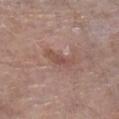Assessment: Captured during whole-body skin photography for melanoma surveillance; the lesion was not biopsied. Clinical summary: The patient is a male about 80 years old. Cropped from a total-body skin-imaging series; the visible field is about 15 mm. On the left lower leg. The lesion's longest dimension is about 3.5 mm. This is a white-light tile.